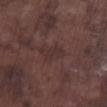Q: Automated lesion metrics?
A: a mean CIELAB color near L≈31 a*≈17 b*≈16, about 5 CIELAB-L* units darker than the surrounding skin, and a normalized lesion–skin contrast near 5; an automated nevus-likeness rating near 0 out of 100 and a detector confidence of about 85 out of 100 that the crop contains a lesion
Q: Lesion location?
A: the right lower leg
Q: Patient demographics?
A: male, aged 73–77
Q: How was this image acquired?
A: 15 mm crop, total-body photography
Q: How large is the lesion?
A: ≈2.5 mm